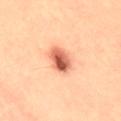* follow-up — imaged on a skin check; not biopsied
* anatomic site — the right thigh
* patient — female, aged 28–32
* acquisition — total-body-photography crop, ~15 mm field of view